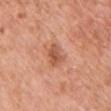{
  "biopsy_status": "not biopsied; imaged during a skin examination",
  "automated_metrics": {
    "nevus_likeness_0_100": 10
  },
  "lesion_size": {
    "long_diameter_mm_approx": 2.5
  },
  "site": "chest",
  "image": {
    "source": "total-body photography crop",
    "field_of_view_mm": 15
  },
  "patient": {
    "sex": "female",
    "age_approx": 40
  },
  "lighting": "white-light"
}